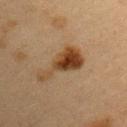Imaged during a routine full-body skin examination; the lesion was not biopsied and no histopathology is available. The lesion is on the left upper arm. Longest diameter approximately 6.5 mm. The subject is a female roughly 40 years of age. A 15 mm crop from a total-body photograph taken for skin-cancer surveillance. This is a cross-polarized tile.